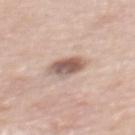Case summary:
- acquisition · total-body-photography crop, ~15 mm field of view
- lesion diameter · ~3.5 mm (longest diameter)
- body site · the upper back
- subject · female, aged 63 to 67
- lighting · white-light illumination